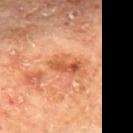{"biopsy_status": "not biopsied; imaged during a skin examination", "patient": {"sex": "male", "age_approx": 65}, "lesion_size": {"long_diameter_mm_approx": 4.5}, "automated_metrics": {"border_irregularity_0_10": 4.5, "peripheral_color_asymmetry": 2.0}, "site": "mid back", "image": {"source": "total-body photography crop", "field_of_view_mm": 15}}